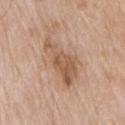A 15 mm crop from a total-body photograph taken for skin-cancer surveillance. Located on the upper back. The subject is a male about 80 years old. The lesion-visualizer software estimated an area of roughly 14 mm², a shape eccentricity near 0.9, and a symmetry-axis asymmetry near 0.4. The software also gave a detector confidence of about 100 out of 100 that the crop contains a lesion.